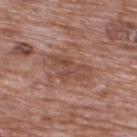Assessment:
Recorded during total-body skin imaging; not selected for excision or biopsy.
Background:
Located on the upper back. Captured under white-light illumination. A roughly 15 mm field-of-view crop from a total-body skin photograph. Measured at roughly 5 mm in maximum diameter. A male patient roughly 70 years of age. Automated tile analysis of the lesion measured an outline eccentricity of about 0.85 (0 = round, 1 = elongated) and two-axis asymmetry of about 0.3. It also reported border irregularity of about 4 on a 0–10 scale and radial color variation of about 1. The software also gave a classifier nevus-likeness of about 0/100 and lesion-presence confidence of about 100/100.